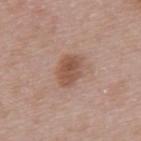This lesion was catalogued during total-body skin photography and was not selected for biopsy. The recorded lesion diameter is about 3.5 mm. A female subject, about 30 years old. The lesion-visualizer software estimated a shape eccentricity near 0.75 and two-axis asymmetry of about 0.2. It also reported lesion-presence confidence of about 100/100. Captured under white-light illumination. A 15 mm crop from a total-body photograph taken for skin-cancer surveillance. The lesion is located on the back.